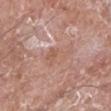{
  "biopsy_status": "not biopsied; imaged during a skin examination",
  "automated_metrics": {
    "area_mm2_approx": 5.0,
    "eccentricity": 0.8,
    "shape_asymmetry": 0.55,
    "cielab_L": 57,
    "cielab_a": 21,
    "cielab_b": 28,
    "vs_skin_contrast_norm": 4.5,
    "border_irregularity_0_10": 6.0,
    "color_variation_0_10": 3.0
  },
  "image": {
    "source": "total-body photography crop",
    "field_of_view_mm": 15
  },
  "site": "right lower leg",
  "patient": {
    "sex": "male",
    "age_approx": 60
  },
  "lesion_size": {
    "long_diameter_mm_approx": 3.5
  }
}